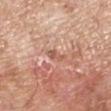No biopsy was performed on this lesion — it was imaged during a full skin examination and was not determined to be concerning.
The lesion-visualizer software estimated an outline eccentricity of about 0.85 (0 = round, 1 = elongated) and a shape-asymmetry score of about 0.4 (0 = symmetric). The software also gave a border-irregularity index near 4/10 and a within-lesion color-variation index near 1.5/10.
The tile uses white-light illumination.
A male patient, about 80 years old.
A lesion tile, about 15 mm wide, cut from a 3D total-body photograph.
The lesion is on the leg.
The lesion's longest dimension is about 2.5 mm.